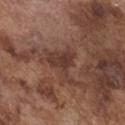{"biopsy_status": "not biopsied; imaged during a skin examination", "patient": {"sex": "male", "age_approx": 75}, "lighting": "white-light", "site": "chest", "lesion_size": {"long_diameter_mm_approx": 5.0}, "image": {"source": "total-body photography crop", "field_of_view_mm": 15}}